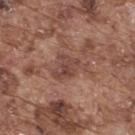Clinical impression: Imaged during a routine full-body skin examination; the lesion was not biopsied and no histopathology is available. Context: The lesion is on the upper back. Imaged with white-light lighting. Cropped from a total-body skin-imaging series; the visible field is about 15 mm. Approximately 4 mm at its widest. A male patient, roughly 75 years of age.